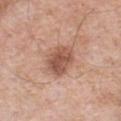Assessment: Imaged during a routine full-body skin examination; the lesion was not biopsied and no histopathology is available. Image and clinical context: The patient is a male aged 63 to 67. This is a white-light tile. From the front of the torso. Approximately 4.5 mm at its widest. Cropped from a whole-body photographic skin survey; the tile spans about 15 mm.